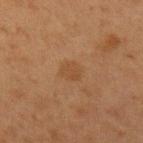Recorded during total-body skin imaging; not selected for excision or biopsy. A region of skin cropped from a whole-body photographic capture, roughly 15 mm wide. From the arm. The patient is a female aged 38–42.